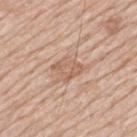notes: no biopsy performed (imaged during a skin exam) | lighting: white-light | subject: male, about 80 years old | acquisition: ~15 mm crop, total-body skin-cancer survey | site: the mid back.